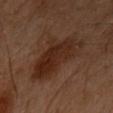Case summary:
- notes: catalogued during a skin exam; not biopsied
- patient: female, aged 58 to 62
- site: the arm
- imaging modality: 15 mm crop, total-body photography